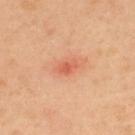| key | value |
|---|---|
| biopsy status | no biopsy performed (imaged during a skin exam) |
| image source | ~15 mm crop, total-body skin-cancer survey |
| diameter | about 2.5 mm |
| subject | female, approximately 40 years of age |
| body site | the upper back |